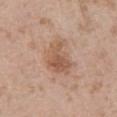Recorded during total-body skin imaging; not selected for excision or biopsy.
The lesion-visualizer software estimated a normalized lesion–skin contrast near 6.5. The analysis additionally found a lesion-detection confidence of about 100/100.
Located on the abdomen.
Cropped from a whole-body photographic skin survey; the tile spans about 15 mm.
Approximately 4.5 mm at its widest.
This is a white-light tile.
A male subject aged around 55.